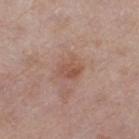Recorded during total-body skin imaging; not selected for excision or biopsy. A 15 mm crop from a total-body photograph taken for skin-cancer surveillance. A female patient aged around 60. The lesion is on the left thigh. Captured under white-light illumination. Longest diameter approximately 3 mm. Automated tile analysis of the lesion measured a mean CIELAB color near L≈52 a*≈21 b*≈27 and roughly 8 lightness units darker than nearby skin. And it measured border irregularity of about 3 on a 0–10 scale. And it measured an automated nevus-likeness rating near 0 out of 100 and a detector confidence of about 100 out of 100 that the crop contains a lesion.